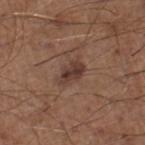Q: Is there a histopathology result?
A: imaged on a skin check; not biopsied
Q: What kind of image is this?
A: total-body-photography crop, ~15 mm field of view
Q: Illumination type?
A: white-light
Q: Lesion size?
A: about 3 mm
Q: Who is the patient?
A: male, about 60 years old
Q: Lesion location?
A: the right lower leg